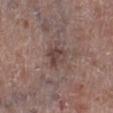– follow-up · total-body-photography surveillance lesion; no biopsy
– subject · male, about 65 years old
– location · the right lower leg
– lighting · white-light
– automated lesion analysis · a lesion area of about 4.5 mm²
– acquisition · ~15 mm tile from a whole-body skin photo